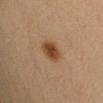Q: Was this lesion biopsied?
A: imaged on a skin check; not biopsied
Q: Who is the patient?
A: female, approximately 30 years of age
Q: Lesion size?
A: ≈3 mm
Q: What is the imaging modality?
A: total-body-photography crop, ~15 mm field of view
Q: Illumination type?
A: cross-polarized illumination
Q: Lesion location?
A: the left upper arm